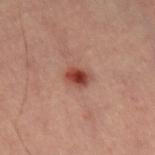subject = female, in their mid-40s
imaging modality = ~15 mm tile from a whole-body skin photo
site = the left thigh